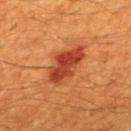No biopsy was performed on this lesion — it was imaged during a full skin examination and was not determined to be concerning. A 15 mm crop from a total-body photograph taken for skin-cancer surveillance. The subject is a male aged approximately 60. An algorithmic analysis of the crop reported an area of roughly 12 mm², a shape eccentricity near 0.85, and a shape-asymmetry score of about 0.2 (0 = symmetric). The analysis additionally found a border-irregularity rating of about 2.5/10, a within-lesion color-variation index near 5/10, and radial color variation of about 1.5. And it measured a classifier nevus-likeness of about 95/100. The lesion is on the mid back.